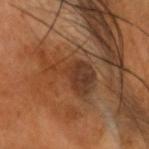Longest diameter approximately 7 mm. Captured under cross-polarized illumination. The lesion is located on the head or neck. A 15 mm close-up tile from a total-body photography series done for melanoma screening. The subject is a male roughly 60 years of age. Automated tile analysis of the lesion measured a lesion area of about 13 mm², an outline eccentricity of about 0.85 (0 = round, 1 = elongated), and a symmetry-axis asymmetry near 0.55. It also reported internal color variation of about 4.5 on a 0–10 scale and a peripheral color-asymmetry measure near 1.5. The software also gave an automated nevus-likeness rating near 0 out of 100 and a lesion-detection confidence of about 95/100.